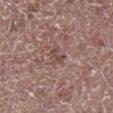Captured during whole-body skin photography for melanoma surveillance; the lesion was not biopsied.
Automated tile analysis of the lesion measured a lesion color around L≈46 a*≈19 b*≈21 in CIELAB, a lesion–skin lightness drop of about 8, and a normalized border contrast of about 6. And it measured a classifier nevus-likeness of about 0/100 and a detector confidence of about 100 out of 100 that the crop contains a lesion.
A lesion tile, about 15 mm wide, cut from a 3D total-body photograph.
The lesion is located on the left lower leg.
The patient is a male in their 70s.